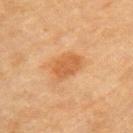Assessment:
The lesion was photographed on a routine skin check and not biopsied; there is no pathology result.
Background:
A lesion tile, about 15 mm wide, cut from a 3D total-body photograph. The lesion is on the left upper arm. A female patient, approximately 80 years of age. The tile uses cross-polarized illumination. Measured at roughly 4 mm in maximum diameter.